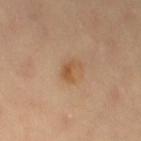workup = catalogued during a skin exam; not biopsied
imaging modality = 15 mm crop, total-body photography
tile lighting = cross-polarized illumination
location = the left thigh
subject = female, about 70 years old